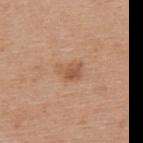<tbp_lesion>
  <biopsy_status>not biopsied; imaged during a skin examination</biopsy_status>
  <site>upper back</site>
  <lesion_size>
    <long_diameter_mm_approx>3.0</long_diameter_mm_approx>
  </lesion_size>
  <lighting>white-light</lighting>
  <automated_metrics>
    <vs_skin_darker_L>9.0</vs_skin_darker_L>
    <vs_skin_contrast_norm>6.5</vs_skin_contrast_norm>
  </automated_metrics>
  <image>
    <source>total-body photography crop</source>
    <field_of_view_mm>15</field_of_view_mm>
  </image>
  <patient>
    <sex>female</sex>
    <age_approx>65</age_approx>
  </patient>
</tbp_lesion>